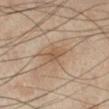  biopsy_status: not biopsied; imaged during a skin examination
  site: right lower leg
  patient:
    sex: male
    age_approx: 55
  lesion_size:
    long_diameter_mm_approx: 3.5
  lighting: cross-polarized
  image:
    source: total-body photography crop
    field_of_view_mm: 15